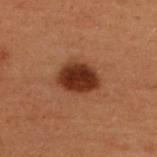workup = total-body-photography surveillance lesion; no biopsy | image = ~15 mm tile from a whole-body skin photo | subject = female, aged approximately 50 | size = ~4.5 mm (longest diameter) | anatomic site = the upper back | illumination = cross-polarized.